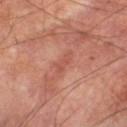Captured during whole-body skin photography for melanoma surveillance; the lesion was not biopsied. A lesion tile, about 15 mm wide, cut from a 3D total-body photograph. Longest diameter approximately 3 mm. On the left thigh. Captured under cross-polarized illumination. A male patient in their 70s. Automated image analysis of the tile measured an outline eccentricity of about 0.9 (0 = round, 1 = elongated) and a symmetry-axis asymmetry near 0.25. It also reported a classifier nevus-likeness of about 0/100 and lesion-presence confidence of about 100/100.